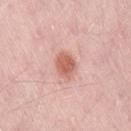* workup: imaged on a skin check; not biopsied
* body site: the right thigh
* tile lighting: white-light illumination
* patient: male, about 50 years old
* imaging modality: 15 mm crop, total-body photography
* TBP lesion metrics: a footprint of about 6 mm² and two-axis asymmetry of about 0.2; a border-irregularity index near 1.5/10, internal color variation of about 3 on a 0–10 scale, and a peripheral color-asymmetry measure near 1; a classifier nevus-likeness of about 90/100 and a lesion-detection confidence of about 100/100
* diameter: ~3.5 mm (longest diameter)